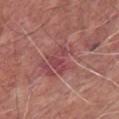lighting: white-light illumination
location: the front of the torso
subject: male, roughly 60 years of age
image: total-body-photography crop, ~15 mm field of view